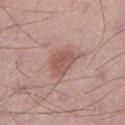The lesion was tiled from a total-body skin photograph and was not biopsied.